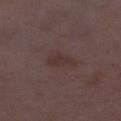Clinical impression: No biopsy was performed on this lesion — it was imaged during a full skin examination and was not determined to be concerning. Context: A 15 mm close-up extracted from a 3D total-body photography capture. Imaged with white-light lighting. An algorithmic analysis of the crop reported a footprint of about 5 mm², an eccentricity of roughly 0.9, and a symmetry-axis asymmetry near 0.35. The software also gave a lesion color around L≈32 a*≈16 b*≈17 in CIELAB and about 5 CIELAB-L* units darker than the surrounding skin. And it measured an automated nevus-likeness rating near 0 out of 100 and a lesion-detection confidence of about 100/100. Located on the left lower leg. A female subject approximately 30 years of age.